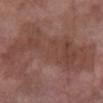Q: How was this image acquired?
A: total-body-photography crop, ~15 mm field of view
Q: What is the anatomic site?
A: the right forearm
Q: What lighting was used for the tile?
A: white-light illumination
Q: What is the lesion's diameter?
A: ≈13.5 mm
Q: What are the patient's age and sex?
A: female, about 70 years old